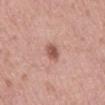Recorded during total-body skin imaging; not selected for excision or biopsy. The lesion is on the mid back. Captured under white-light illumination. The lesion-visualizer software estimated an average lesion color of about L≈54 a*≈24 b*≈27 (CIELAB) and a normalized border contrast of about 8.5. The analysis additionally found a border-irregularity index near 2.5/10, internal color variation of about 2 on a 0–10 scale, and peripheral color asymmetry of about 0.5. The software also gave a classifier nevus-likeness of about 95/100 and a lesion-detection confidence of about 100/100. Approximately 2.5 mm at its widest. A male subject, about 55 years old. A lesion tile, about 15 mm wide, cut from a 3D total-body photograph.